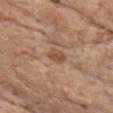Captured during whole-body skin photography for melanoma surveillance; the lesion was not biopsied.
The lesion is on the front of the torso.
This image is a 15 mm lesion crop taken from a total-body photograph.
The subject is a male aged around 85 or older.